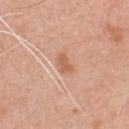Assessment: The lesion was tiled from a total-body skin photograph and was not biopsied. Image and clinical context: Automated tile analysis of the lesion measured border irregularity of about 2.5 on a 0–10 scale, internal color variation of about 1.5 on a 0–10 scale, and radial color variation of about 0.5. It also reported a nevus-likeness score of about 45/100 and lesion-presence confidence of about 100/100. The recorded lesion diameter is about 2.5 mm. A 15 mm close-up tile from a total-body photography series done for melanoma screening. On the chest. A male subject, aged around 50. Captured under white-light illumination.